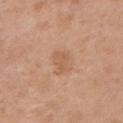Clinical impression: Captured during whole-body skin photography for melanoma surveillance; the lesion was not biopsied. Background: About 2.5 mm across. A 15 mm close-up extracted from a 3D total-body photography capture. A female patient, about 40 years old. An algorithmic analysis of the crop reported a footprint of about 4.5 mm², a shape eccentricity near 0.75, and two-axis asymmetry of about 0.3. The analysis additionally found border irregularity of about 3 on a 0–10 scale, a within-lesion color-variation index near 1.5/10, and radial color variation of about 0.5. The lesion is on the left upper arm.